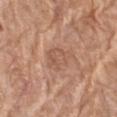notes: imaged on a skin check; not biopsied
acquisition: ~15 mm tile from a whole-body skin photo
illumination: white-light illumination
site: the left thigh
image-analysis metrics: a footprint of about 7.5 mm² and a symmetry-axis asymmetry near 0.35; a classifier nevus-likeness of about 0/100
patient: female, approximately 75 years of age